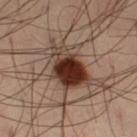<tbp_lesion>
<biopsy_status>not biopsied; imaged during a skin examination</biopsy_status>
<lesion_size>
  <long_diameter_mm_approx>8.5</long_diameter_mm_approx>
</lesion_size>
<patient>
  <sex>male</sex>
  <age_approx>40</age_approx>
</patient>
<image>
  <source>total-body photography crop</source>
  <field_of_view_mm>15</field_of_view_mm>
</image>
<site>left thigh</site>
<lighting>cross-polarized</lighting>
</tbp_lesion>